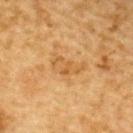Findings:
• automated metrics · a lesion area of about 6 mm² and two-axis asymmetry of about 0.4; a lesion color around L≈51 a*≈19 b*≈39 in CIELAB and roughly 7 lightness units darker than nearby skin
• image · ~15 mm crop, total-body skin-cancer survey
• subject · male, aged around 85 or older
• size · ≈4 mm
• illumination · cross-polarized
• body site · the upper back